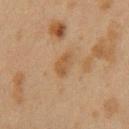Findings:
- biopsy status · catalogued during a skin exam; not biopsied
- patient · female, aged 38–42
- body site · the left upper arm
- lighting · cross-polarized illumination
- acquisition · ~15 mm tile from a whole-body skin photo